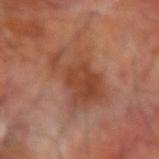- biopsy status · catalogued during a skin exam; not biopsied
- image source · ~15 mm tile from a whole-body skin photo
- site · the right forearm
- diameter · ≈7 mm
- image-analysis metrics · a border-irregularity rating of about 7/10
- patient · male, aged around 70
- tile lighting · cross-polarized